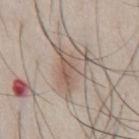- follow-up: total-body-photography surveillance lesion; no biopsy
- illumination: white-light
- anatomic site: the front of the torso
- size: ≈5 mm
- image: total-body-photography crop, ~15 mm field of view
- TBP lesion metrics: a footprint of about 12 mm², an outline eccentricity of about 0.65 (0 = round, 1 = elongated), and a shape-asymmetry score of about 0.5 (0 = symmetric); a border-irregularity index near 6.5/10, internal color variation of about 5.5 on a 0–10 scale, and a peripheral color-asymmetry measure near 2; an automated nevus-likeness rating near 5 out of 100 and a lesion-detection confidence of about 75/100
- subject: male, roughly 45 years of age